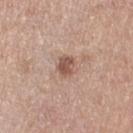Case summary:
– biopsy status — no biopsy performed (imaged during a skin exam)
– size — about 2.5 mm
– imaging modality — ~15 mm crop, total-body skin-cancer survey
– tile lighting — white-light illumination
– TBP lesion metrics — a footprint of about 4.5 mm², an eccentricity of roughly 0.75, and a symmetry-axis asymmetry near 0.25; a mean CIELAB color near L≈53 a*≈20 b*≈26, a lesion–skin lightness drop of about 12, and a lesion-to-skin contrast of about 8 (normalized; higher = more distinct)
– subject — female, about 40 years old
– location — the leg